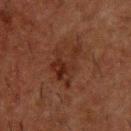Impression: The lesion was photographed on a routine skin check and not biopsied; there is no pathology result. Background: A close-up tile cropped from a whole-body skin photograph, about 15 mm across. Longest diameter approximately 5 mm. The lesion is on the upper back. Automated tile analysis of the lesion measured an area of roughly 9.5 mm² and an eccentricity of roughly 0.7. The software also gave a lesion color around L≈22 a*≈18 b*≈22 in CIELAB, roughly 6 lightness units darker than nearby skin, and a normalized lesion–skin contrast near 7. The software also gave border irregularity of about 9 on a 0–10 scale and peripheral color asymmetry of about 1.5. The subject is a male roughly 50 years of age. The tile uses cross-polarized illumination.